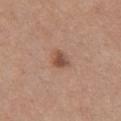Assessment: The lesion was tiled from a total-body skin photograph and was not biopsied. Context: Longest diameter approximately 2.5 mm. A region of skin cropped from a whole-body photographic capture, roughly 15 mm wide. Captured under white-light illumination. The lesion-visualizer software estimated an area of roughly 4.5 mm², a shape eccentricity near 0.5, and a symmetry-axis asymmetry near 0.25. The analysis additionally found an average lesion color of about L≈51 a*≈21 b*≈29 (CIELAB), roughly 10 lightness units darker than nearby skin, and a lesion-to-skin contrast of about 7.5 (normalized; higher = more distinct). A female subject aged approximately 55. On the front of the torso.